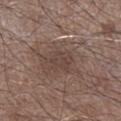Captured during whole-body skin photography for melanoma surveillance; the lesion was not biopsied. A 15 mm close-up tile from a total-body photography series done for melanoma screening. The patient is a male aged around 60. Imaged with white-light lighting. The lesion's longest dimension is about 3.5 mm. The total-body-photography lesion software estimated a lesion area of about 6 mm², a shape eccentricity near 0.7, and a shape-asymmetry score of about 0.35 (0 = symmetric). The analysis additionally found a color-variation rating of about 3/10 and peripheral color asymmetry of about 1. On the right lower leg.